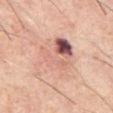Q: Was this lesion biopsied?
A: imaged on a skin check; not biopsied
Q: Patient demographics?
A: male, aged 58–62
Q: How was this image acquired?
A: ~15 mm crop, total-body skin-cancer survey
Q: Lesion location?
A: the abdomen